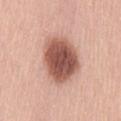biopsy_status: not biopsied; imaged during a skin examination
patient:
  sex: male
  age_approx: 50
lighting: white-light
lesion_size:
  long_diameter_mm_approx: 6.0
automated_metrics:
  area_mm2_approx: 22.0
  eccentricity: 0.6
  shape_asymmetry: 0.15
  vs_skin_contrast_norm: 11.5
image:
  source: total-body photography crop
  field_of_view_mm: 15
site: lower back